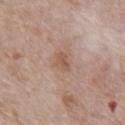Case summary:
* biopsy status — catalogued during a skin exam; not biopsied
* patient — male, aged 68 to 72
* imaging modality — total-body-photography crop, ~15 mm field of view
* site — the abdomen